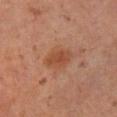Recorded during total-body skin imaging; not selected for excision or biopsy. An algorithmic analysis of the crop reported a lesion color around L≈44 a*≈23 b*≈32 in CIELAB and roughly 7 lightness units darker than nearby skin. The software also gave a nevus-likeness score of about 55/100. The subject is a male about 60 years old. A 15 mm close-up tile from a total-body photography series done for melanoma screening. The lesion is located on the right lower leg. The tile uses cross-polarized illumination. About 4 mm across.